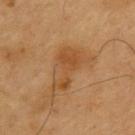Captured under cross-polarized illumination. A close-up tile cropped from a whole-body skin photograph, about 15 mm across. An algorithmic analysis of the crop reported a mean CIELAB color near L≈49 a*≈21 b*≈39, a lesion–skin lightness drop of about 7, and a lesion-to-skin contrast of about 6 (normalized; higher = more distinct). It also reported a border-irregularity rating of about 5.5/10, internal color variation of about 5 on a 0–10 scale, and a peripheral color-asymmetry measure near 1.5. Located on the upper back. Longest diameter approximately 6 mm. The subject is a male aged around 60.